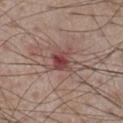<lesion>
<biopsy_status>not biopsied; imaged during a skin examination</biopsy_status>
</lesion>